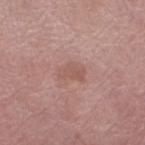Clinical impression:
No biopsy was performed on this lesion — it was imaged during a full skin examination and was not determined to be concerning.
Acquisition and patient details:
Automated image analysis of the tile measured a border-irregularity rating of about 3.5/10 and a peripheral color-asymmetry measure near 0.5. It also reported a nevus-likeness score of about 0/100 and a detector confidence of about 100 out of 100 that the crop contains a lesion. Imaged with white-light lighting. The lesion is located on the right lower leg. About 2.5 mm across. A female patient, aged around 70. A 15 mm close-up extracted from a 3D total-body photography capture.